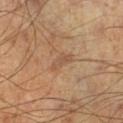The lesion was photographed on a routine skin check and not biopsied; there is no pathology result. The total-body-photography lesion software estimated a lesion color around L≈52 a*≈19 b*≈32 in CIELAB, roughly 7 lightness units darker than nearby skin, and a normalized border contrast of about 5. The software also gave a nevus-likeness score of about 0/100 and a lesion-detection confidence of about 100/100. A male subject aged 43 to 47. The lesion is located on the left lower leg. Longest diameter approximately 3 mm. A roughly 15 mm field-of-view crop from a total-body skin photograph.